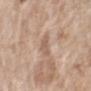lesion diameter: ≈2.5 mm | body site: the left forearm | patient: female, about 75 years old | acquisition: 15 mm crop, total-body photography | lighting: white-light.